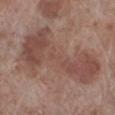Impression: Captured during whole-body skin photography for melanoma surveillance; the lesion was not biopsied. Image and clinical context: The subject is a male about 70 years old. Approximately 11 mm at its widest. A 15 mm crop from a total-body photograph taken for skin-cancer surveillance. Captured under white-light illumination. Automated image analysis of the tile measured a lesion area of about 34 mm² and a shape eccentricity near 0.9. From the left lower leg.